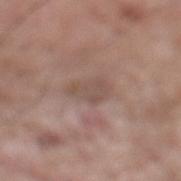The lesion was photographed on a routine skin check and not biopsied; there is no pathology result. On the mid back. Imaged with white-light lighting. The lesion-visualizer software estimated a lesion area of about 6 mm², an outline eccentricity of about 0.8 (0 = round, 1 = elongated), and a shape-asymmetry score of about 0.3 (0 = symmetric). The analysis additionally found a classifier nevus-likeness of about 0/100. A close-up tile cropped from a whole-body skin photograph, about 15 mm across. The subject is a male aged 58 to 62. The recorded lesion diameter is about 3.5 mm.